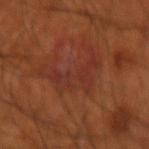Clinical impression: This lesion was catalogued during total-body skin photography and was not selected for biopsy. Acquisition and patient details: A male patient roughly 55 years of age. This is a cross-polarized tile. The lesion is on the right forearm. Longest diameter approximately 7 mm. Cropped from a whole-body photographic skin survey; the tile spans about 15 mm.